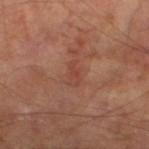<case>
  <biopsy_status>not biopsied; imaged during a skin examination</biopsy_status>
  <automated_metrics>
    <area_mm2_approx>3.5</area_mm2_approx>
    <eccentricity>0.85</eccentricity>
    <shape_asymmetry>0.4</shape_asymmetry>
    <border_irregularity_0_10>4.5</border_irregularity_0_10>
    <color_variation_0_10>2.0</color_variation_0_10>
    <peripheral_color_asymmetry>0.5</peripheral_color_asymmetry>
  </automated_metrics>
  <image>
    <source>total-body photography crop</source>
    <field_of_view_mm>15</field_of_view_mm>
  </image>
  <lesion_size>
    <long_diameter_mm_approx>3.0</long_diameter_mm_approx>
  </lesion_size>
  <lighting>cross-polarized</lighting>
  <patient>
    <sex>male</sex>
    <age_approx>70</age_approx>
  </patient>
  <site>right thigh</site>
</case>